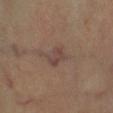biopsy status: imaged on a skin check; not biopsied
body site: the right lower leg
subject: male, roughly 55 years of age
illumination: cross-polarized
imaging modality: total-body-photography crop, ~15 mm field of view
lesion diameter: about 2.5 mm